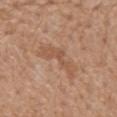Imaged during a routine full-body skin examination; the lesion was not biopsied and no histopathology is available. Automated image analysis of the tile measured an outline eccentricity of about 0.9 (0 = round, 1 = elongated) and a shape-asymmetry score of about 0.7 (0 = symmetric). The software also gave a border-irregularity index near 9/10. It also reported an automated nevus-likeness rating near 0 out of 100. A 15 mm close-up extracted from a 3D total-body photography capture. The lesion is on the back. The tile uses white-light illumination. Approximately 5 mm at its widest. The subject is a male aged 68 to 72.